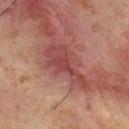follow-up = imaged on a skin check; not biopsied
location = the back
subject = male, aged around 65
imaging modality = ~15 mm tile from a whole-body skin photo
lighting = cross-polarized